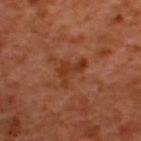<case>
<biopsy_status>not biopsied; imaged during a skin examination</biopsy_status>
<site>back</site>
<lighting>cross-polarized</lighting>
<image>
  <source>total-body photography crop</source>
  <field_of_view_mm>15</field_of_view_mm>
</image>
<patient>
  <sex>male</sex>
  <age_approx>50</age_approx>
</patient>
</case>